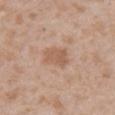Part of a total-body skin-imaging series; this lesion was reviewed on a skin check and was not flagged for biopsy.
Captured under white-light illumination.
Automated tile analysis of the lesion measured a lesion area of about 6 mm², an outline eccentricity of about 0.7 (0 = round, 1 = elongated), and two-axis asymmetry of about 0.25. The analysis additionally found a classifier nevus-likeness of about 0/100 and a detector confidence of about 100 out of 100 that the crop contains a lesion.
From the abdomen.
A male subject, in their mid-60s.
A region of skin cropped from a whole-body photographic capture, roughly 15 mm wide.